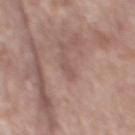biopsy status=catalogued during a skin exam; not biopsied
image source=15 mm crop, total-body photography
patient=male, aged around 65
anatomic site=the mid back